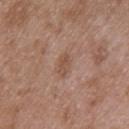{"biopsy_status": "not biopsied; imaged during a skin examination", "image": {"source": "total-body photography crop", "field_of_view_mm": 15}, "lighting": "white-light", "patient": {"sex": "male", "age_approx": 50}, "site": "upper back"}